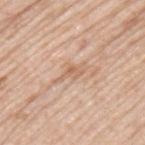The lesion was tiled from a total-body skin photograph and was not biopsied. Imaged with white-light lighting. The subject is a male aged 78–82. Approximately 3 mm at its widest. The lesion is on the upper back. Cropped from a total-body skin-imaging series; the visible field is about 15 mm. The total-body-photography lesion software estimated a mean CIELAB color near L≈63 a*≈19 b*≈32 and a lesion-to-skin contrast of about 6 (normalized; higher = more distinct). The analysis additionally found a border-irregularity index near 6/10, a color-variation rating of about 0/10, and a peripheral color-asymmetry measure near 0. The analysis additionally found an automated nevus-likeness rating near 0 out of 100.